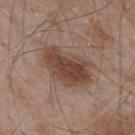Impression: This lesion was catalogued during total-body skin photography and was not selected for biopsy. Context: The total-body-photography lesion software estimated two-axis asymmetry of about 0.2. And it measured an average lesion color of about L≈44 a*≈18 b*≈26 (CIELAB), about 11 CIELAB-L* units darker than the surrounding skin, and a normalized border contrast of about 8.5. It also reported border irregularity of about 2.5 on a 0–10 scale, a color-variation rating of about 5/10, and a peripheral color-asymmetry measure near 1.5. Measured at roughly 6.5 mm in maximum diameter. Captured under white-light illumination. The lesion is located on the upper back. The patient is a male in their mid-50s. This image is a 15 mm lesion crop taken from a total-body photograph.